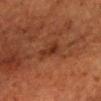This lesion was catalogued during total-body skin photography and was not selected for biopsy. Captured under cross-polarized illumination. The patient is a male aged 63 to 67. On the head or neck. Cropped from a whole-body photographic skin survey; the tile spans about 15 mm. Measured at roughly 3 mm in maximum diameter. An algorithmic analysis of the crop reported a lesion area of about 4 mm², an outline eccentricity of about 0.85 (0 = round, 1 = elongated), and two-axis asymmetry of about 0.4. The software also gave an automated nevus-likeness rating near 10 out of 100.